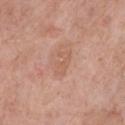workup = no biopsy performed (imaged during a skin exam) | image source = ~15 mm crop, total-body skin-cancer survey | location = the front of the torso | subject = male, roughly 80 years of age.